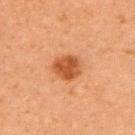No biopsy was performed on this lesion — it was imaged during a full skin examination and was not determined to be concerning.
This is a cross-polarized tile.
A roughly 15 mm field-of-view crop from a total-body skin photograph.
Located on the left upper arm.
A female patient in their 60s.